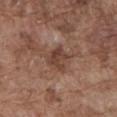Assessment: Imaged during a routine full-body skin examination; the lesion was not biopsied and no histopathology is available. Background: A male patient aged approximately 75. On the front of the torso. A lesion tile, about 15 mm wide, cut from a 3D total-body photograph.